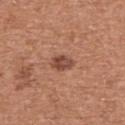Located on the upper back. A lesion tile, about 15 mm wide, cut from a 3D total-body photograph. Longest diameter approximately 2.5 mm. A female patient about 60 years old. Automated tile analysis of the lesion measured a footprint of about 4 mm², an outline eccentricity of about 0.8 (0 = round, 1 = elongated), and a shape-asymmetry score of about 0.2 (0 = symmetric). The analysis additionally found a mean CIELAB color near L≈46 a*≈24 b*≈29, a lesion–skin lightness drop of about 12, and a lesion-to-skin contrast of about 8.5 (normalized; higher = more distinct). The analysis additionally found a nevus-likeness score of about 80/100 and a lesion-detection confidence of about 100/100.